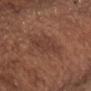Clinical impression: The lesion was photographed on a routine skin check and not biopsied; there is no pathology result. Background: Imaged with white-light lighting. The lesion is located on the chest. The total-body-photography lesion software estimated a lesion color around L≈39 a*≈21 b*≈26 in CIELAB, about 6 CIELAB-L* units darker than the surrounding skin, and a lesion-to-skin contrast of about 5.5 (normalized; higher = more distinct). And it measured a color-variation rating of about 2/10. It also reported an automated nevus-likeness rating near 0 out of 100. Cropped from a total-body skin-imaging series; the visible field is about 15 mm. Measured at roughly 4.5 mm in maximum diameter. A male subject, aged approximately 75.